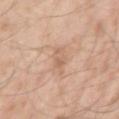biopsy_status: not biopsied; imaged during a skin examination
lighting: white-light
patient:
  sex: male
  age_approx: 35
image:
  source: total-body photography crop
  field_of_view_mm: 15
site: right upper arm
lesion_size:
  long_diameter_mm_approx: 3.5
automated_metrics:
  eccentricity: 0.85
  shape_asymmetry: 0.3
  cielab_L: 63
  cielab_a: 19
  cielab_b: 31
  vs_skin_darker_L: 8.0
  border_irregularity_0_10: 4.0
  color_variation_0_10: 3.5
  peripheral_color_asymmetry: 1.5
  nevus_likeness_0_100: 0
  lesion_detection_confidence_0_100: 100